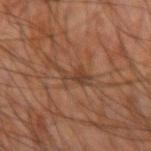Context: A male patient in their mid- to late 60s. On the right forearm. Cropped from a total-body skin-imaging series; the visible field is about 15 mm. This is a cross-polarized tile.